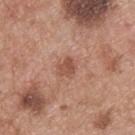Q: Where on the body is the lesion?
A: the upper back
Q: How was this image acquired?
A: 15 mm crop, total-body photography
Q: What lighting was used for the tile?
A: white-light illumination
Q: Patient demographics?
A: male, aged 53–57
Q: What did automated image analysis measure?
A: an average lesion color of about L≈51 a*≈23 b*≈29 (CIELAB), a lesion–skin lightness drop of about 9, and a normalized lesion–skin contrast near 6.5; a nevus-likeness score of about 15/100